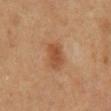Findings:
- notes — no biopsy performed (imaged during a skin exam)
- image — ~15 mm tile from a whole-body skin photo
- patient — male, aged approximately 60
- site — the back